Cropped from a whole-body photographic skin survey; the tile spans about 15 mm; the lesion is on the mid back; a female patient aged 43 to 47.
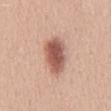{"diagnosis": {"histopathology": "dysplastic (Clark) nevus", "malignancy": "benign", "taxonomic_path": ["Benign", "Benign melanocytic proliferations", "Nevus", "Nevus, Atypical, Dysplastic, or Clark"]}}